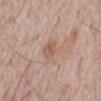Q: Was a biopsy performed?
A: catalogued during a skin exam; not biopsied
Q: Automated lesion metrics?
A: a mean CIELAB color near L≈57 a*≈19 b*≈27 and a normalized lesion–skin contrast near 5.5; a border-irregularity rating of about 1.5/10, a color-variation rating of about 3/10, and a peripheral color-asymmetry measure near 1; a nevus-likeness score of about 5/100 and lesion-presence confidence of about 100/100
Q: What kind of image is this?
A: ~15 mm tile from a whole-body skin photo
Q: Lesion size?
A: about 2.5 mm
Q: Lesion location?
A: the abdomen
Q: Patient demographics?
A: male, in their 70s
Q: What lighting was used for the tile?
A: white-light illumination